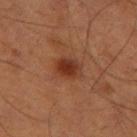The lesion was tiled from a total-body skin photograph and was not biopsied.
A male subject approximately 55 years of age.
Automated tile analysis of the lesion measured an area of roughly 6.5 mm², a shape eccentricity near 0.65, and a shape-asymmetry score of about 0.15 (0 = symmetric). The analysis additionally found a normalized border contrast of about 8.5. And it measured a classifier nevus-likeness of about 95/100 and lesion-presence confidence of about 100/100.
A 15 mm close-up tile from a total-body photography series done for melanoma screening.
The lesion is on the left thigh.